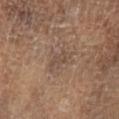{"biopsy_status": "not biopsied; imaged during a skin examination", "image": {"source": "total-body photography crop", "field_of_view_mm": 15}, "automated_metrics": {"cielab_L": 44, "cielab_a": 14, "cielab_b": 24, "vs_skin_darker_L": 6.0, "vs_skin_contrast_norm": 5.0, "border_irregularity_0_10": 4.0, "color_variation_0_10": 0.5, "peripheral_color_asymmetry": 0.0, "nevus_likeness_0_100": 0, "lesion_detection_confidence_0_100": 70}, "patient": {"sex": "male", "age_approx": 65}, "lesion_size": {"long_diameter_mm_approx": 3.0}, "lighting": "cross-polarized", "site": "left lower leg"}